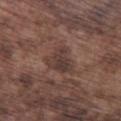{"biopsy_status": "not biopsied; imaged during a skin examination", "image": {"source": "total-body photography crop", "field_of_view_mm": 15}, "lesion_size": {"long_diameter_mm_approx": 4.0}, "site": "leg", "patient": {"sex": "male", "age_approx": 75}, "lighting": "white-light", "automated_metrics": {"area_mm2_approx": 9.5, "eccentricity": 0.55, "shape_asymmetry": 0.4, "border_irregularity_0_10": 4.0, "color_variation_0_10": 4.0, "peripheral_color_asymmetry": 1.5, "nevus_likeness_0_100": 0, "lesion_detection_confidence_0_100": 90}}